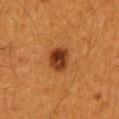notes: no biopsy performed (imaged during a skin exam); tile lighting: cross-polarized illumination; image: total-body-photography crop, ~15 mm field of view; body site: the lower back; subject: male, approximately 60 years of age.